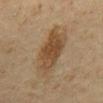This is a cross-polarized tile. A 15 mm close-up extracted from a 3D total-body photography capture. The lesion's longest dimension is about 6 mm. A male subject roughly 60 years of age. Located on the mid back. The lesion-visualizer software estimated a lesion area of about 17 mm², a shape eccentricity near 0.8, and a shape-asymmetry score of about 0.25 (0 = symmetric). The analysis additionally found a lesion color around L≈38 a*≈13 b*≈27 in CIELAB. It also reported border irregularity of about 3.5 on a 0–10 scale, a within-lesion color-variation index near 3/10, and radial color variation of about 1.